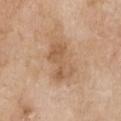Q: Was a biopsy performed?
A: no biopsy performed (imaged during a skin exam)
Q: How was this image acquired?
A: ~15 mm crop, total-body skin-cancer survey
Q: What lighting was used for the tile?
A: white-light illumination
Q: What did automated image analysis measure?
A: a lesion area of about 14 mm², an outline eccentricity of about 0.85 (0 = round, 1 = elongated), and a shape-asymmetry score of about 0.25 (0 = symmetric); lesion-presence confidence of about 100/100
Q: Lesion size?
A: ~5.5 mm (longest diameter)
Q: Lesion location?
A: the chest
Q: What are the patient's age and sex?
A: female, in their mid-60s